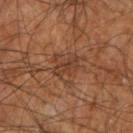Context:
Longest diameter approximately 3.5 mm. A male patient, aged approximately 60. On the right forearm. This is a cross-polarized tile. A lesion tile, about 15 mm wide, cut from a 3D total-body photograph.